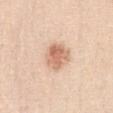follow-up: no biopsy performed (imaged during a skin exam)
TBP lesion metrics: an area of roughly 9 mm², an outline eccentricity of about 0.35 (0 = round, 1 = elongated), and two-axis asymmetry of about 0.2; a color-variation rating of about 4/10 and radial color variation of about 1.5; an automated nevus-likeness rating near 90 out of 100 and a detector confidence of about 100 out of 100 that the crop contains a lesion
patient: female, in their mid- to late 50s
lighting: white-light illumination
diameter: ≈3.5 mm
image source: total-body-photography crop, ~15 mm field of view
body site: the chest